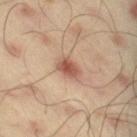Assessment: Imaged during a routine full-body skin examination; the lesion was not biopsied and no histopathology is available. Clinical summary: A male patient aged approximately 45. On the right thigh. Measured at roughly 2.5 mm in maximum diameter. A 15 mm close-up extracted from a 3D total-body photography capture.